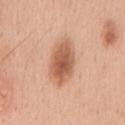Assessment: Part of a total-body skin-imaging series; this lesion was reviewed on a skin check and was not flagged for biopsy. Image and clinical context: On the mid back. Approximately 6 mm at its widest. Captured under white-light illumination. A 15 mm crop from a total-body photograph taken for skin-cancer surveillance. A male subject aged approximately 40.